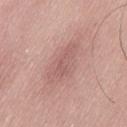{"biopsy_status": "not biopsied; imaged during a skin examination", "lighting": "white-light", "site": "lower back", "image": {"source": "total-body photography crop", "field_of_view_mm": 15}, "automated_metrics": {"area_mm2_approx": 13.0, "eccentricity": 0.9, "cielab_L": 59, "cielab_a": 22, "cielab_b": 23, "vs_skin_darker_L": 7.0, "vs_skin_contrast_norm": 5.0, "color_variation_0_10": 2.5, "peripheral_color_asymmetry": 1.0}, "lesion_size": {"long_diameter_mm_approx": 6.0}, "patient": {"sex": "male", "age_approx": 50}}